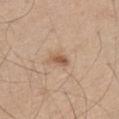Captured during whole-body skin photography for melanoma surveillance; the lesion was not biopsied.
The patient is a male approximately 60 years of age.
The lesion-visualizer software estimated a mean CIELAB color near L≈56 a*≈19 b*≈32 and a lesion-to-skin contrast of about 7.5 (normalized; higher = more distinct). The software also gave a border-irregularity rating of about 3/10, internal color variation of about 1.5 on a 0–10 scale, and a peripheral color-asymmetry measure near 0.5. The software also gave an automated nevus-likeness rating near 70 out of 100 and a lesion-detection confidence of about 100/100.
The tile uses white-light illumination.
A 15 mm crop from a total-body photograph taken for skin-cancer surveillance.
The lesion's longest dimension is about 2.5 mm.
The lesion is located on the abdomen.